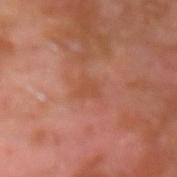notes = catalogued during a skin exam; not biopsied | size = about 2.5 mm | tile lighting = cross-polarized illumination | patient = male, roughly 30 years of age | image-analysis metrics = an eccentricity of roughly 0.65; a lesion color around L≈48 a*≈26 b*≈32 in CIELAB, roughly 5 lightness units darker than nearby skin, and a normalized border contrast of about 4.5 | image source = ~15 mm crop, total-body skin-cancer survey | site = the left forearm.